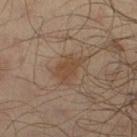biopsy status = catalogued during a skin exam; not biopsied | location = the leg | image source = ~15 mm tile from a whole-body skin photo | patient = male, aged 63 to 67 | diameter = ~3.5 mm (longest diameter) | illumination = cross-polarized.